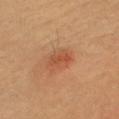biopsy status: imaged on a skin check; not biopsied | location: the head or neck | automated metrics: a lesion color around L≈52 a*≈28 b*≈37 in CIELAB and a lesion-to-skin contrast of about 6 (normalized; higher = more distinct); peripheral color asymmetry of about 1.5; an automated nevus-likeness rating near 80 out of 100 and a detector confidence of about 100 out of 100 that the crop contains a lesion | acquisition: ~15 mm tile from a whole-body skin photo | subject: female, in their 60s.